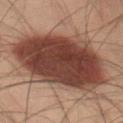Findings:
- lesion size — ≈12.5 mm
- site — the left thigh
- imaging modality — ~15 mm crop, total-body skin-cancer survey
- illumination — cross-polarized
- patient — male, in their 50s